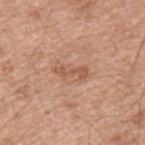• biopsy status — catalogued during a skin exam; not biopsied
• imaging modality — ~15 mm crop, total-body skin-cancer survey
• location — the upper back
• subject — male, approximately 55 years of age
• size — ≈3.5 mm